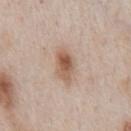Q: Was a biopsy performed?
A: total-body-photography surveillance lesion; no biopsy